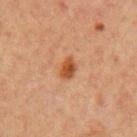• subject · female, about 40 years old
• image-analysis metrics · a footprint of about 3.5 mm² and a shape eccentricity near 0.75; a mean CIELAB color near L≈42 a*≈23 b*≈34, roughly 10 lightness units darker than nearby skin, and a lesion-to-skin contrast of about 9 (normalized; higher = more distinct); a border-irregularity index near 2/10, a color-variation rating of about 2.5/10, and peripheral color asymmetry of about 0.5; a nevus-likeness score of about 95/100
• location · the left upper arm
• size · about 2.5 mm
• image source · total-body-photography crop, ~15 mm field of view
• tile lighting · cross-polarized illumination